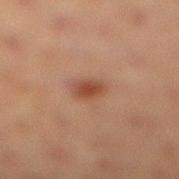Recorded during total-body skin imaging; not selected for excision or biopsy. A close-up tile cropped from a whole-body skin photograph, about 15 mm across. Located on the right lower leg. A female patient, aged approximately 40. The recorded lesion diameter is about 2.5 mm. The total-body-photography lesion software estimated a border-irregularity index near 1/10 and a within-lesion color-variation index near 2.5/10. And it measured an automated nevus-likeness rating near 90 out of 100 and lesion-presence confidence of about 100/100. This is a cross-polarized tile.